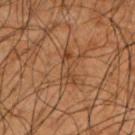Part of a total-body skin-imaging series; this lesion was reviewed on a skin check and was not flagged for biopsy. A male patient, approximately 50 years of age. Imaged with cross-polarized lighting. A region of skin cropped from a whole-body photographic capture, roughly 15 mm wide. From the left upper arm. The lesion's longest dimension is about 3.5 mm.